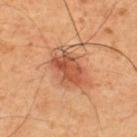lesion_size:
  long_diameter_mm_approx: 5.5
image:
  source: total-body photography crop
  field_of_view_mm: 15
site: upper back
patient:
  sex: male
  age_approx: 50
automated_metrics:
  cielab_L: 52
  cielab_a: 26
  cielab_b: 34
  vs_skin_darker_L: 10.0
  vs_skin_contrast_norm: 7.5
lighting: cross-polarized